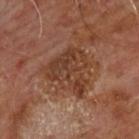Recorded during total-body skin imaging; not selected for excision or biopsy.
About 5.5 mm across.
From the chest.
An algorithmic analysis of the crop reported a footprint of about 16 mm², an outline eccentricity of about 0.45 (0 = round, 1 = elongated), and a shape-asymmetry score of about 0.5 (0 = symmetric). It also reported a lesion color around L≈36 a*≈20 b*≈28 in CIELAB, a lesion–skin lightness drop of about 8, and a normalized border contrast of about 7.5. And it measured a border-irregularity index near 6/10 and a color-variation rating of about 4.5/10.
The tile uses cross-polarized illumination.
A roughly 15 mm field-of-view crop from a total-body skin photograph.
The patient is a male aged around 60.